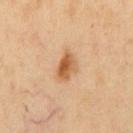notes — catalogued during a skin exam; not biopsied | size — ~3.5 mm (longest diameter) | subject — male, roughly 50 years of age | anatomic site — the chest | illumination — cross-polarized | imaging modality — ~15 mm tile from a whole-body skin photo.